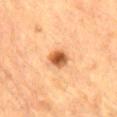Clinical impression: Part of a total-body skin-imaging series; this lesion was reviewed on a skin check and was not flagged for biopsy. Acquisition and patient details: About 2.5 mm across. A male patient, aged 83 or over. Cropped from a total-body skin-imaging series; the visible field is about 15 mm. The tile uses cross-polarized illumination. On the back.